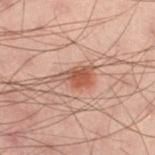Imaged during a routine full-body skin examination; the lesion was not biopsied and no histopathology is available. Measured at roughly 4 mm in maximum diameter. A male subject aged approximately 40. Imaged with cross-polarized lighting. A 15 mm close-up tile from a total-body photography series done for melanoma screening. The lesion is located on the left thigh. Automated tile analysis of the lesion measured an area of roughly 7 mm², an outline eccentricity of about 0.8 (0 = round, 1 = elongated), and two-axis asymmetry of about 0.4. The software also gave a lesion color around L≈43 a*≈20 b*≈25 in CIELAB and a lesion–skin lightness drop of about 9. The software also gave border irregularity of about 4.5 on a 0–10 scale, a color-variation rating of about 3/10, and radial color variation of about 1. The analysis additionally found a classifier nevus-likeness of about 95/100.